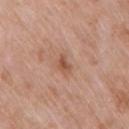No biopsy was performed on this lesion — it was imaged during a full skin examination and was not determined to be concerning. The lesion is located on the right upper arm. A lesion tile, about 15 mm wide, cut from a 3D total-body photograph. A female subject, about 70 years old.